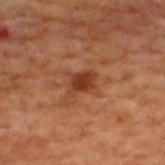Assessment: No biopsy was performed on this lesion — it was imaged during a full skin examination and was not determined to be concerning. Image and clinical context: This is a cross-polarized tile. The subject is a male approximately 70 years of age. From the upper back. Longest diameter approximately 3 mm. This image is a 15 mm lesion crop taken from a total-body photograph.